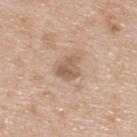Captured during whole-body skin photography for melanoma surveillance; the lesion was not biopsied. From the upper back. The tile uses white-light illumination. This image is a 15 mm lesion crop taken from a total-body photograph. The total-body-photography lesion software estimated about 10 CIELAB-L* units darker than the surrounding skin and a lesion-to-skin contrast of about 6.5 (normalized; higher = more distinct). The lesion's longest dimension is about 3.5 mm. A male patient aged around 65.